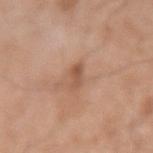No biopsy was performed on this lesion — it was imaged during a full skin examination and was not determined to be concerning.
A male subject, aged 53–57.
Longest diameter approximately 2.5 mm.
Captured under white-light illumination.
The total-body-photography lesion software estimated an area of roughly 3 mm², an outline eccentricity of about 0.8 (0 = round, 1 = elongated), and two-axis asymmetry of about 0.25. It also reported a border-irregularity index near 2.5/10, internal color variation of about 3 on a 0–10 scale, and radial color variation of about 1.
A close-up tile cropped from a whole-body skin photograph, about 15 mm across.
On the right upper arm.